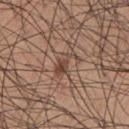Part of a total-body skin-imaging series; this lesion was reviewed on a skin check and was not flagged for biopsy. Imaged with white-light lighting. A region of skin cropped from a whole-body photographic capture, roughly 15 mm wide. The patient is a male aged around 55. Longest diameter approximately 3.5 mm. The lesion is on the left thigh. An algorithmic analysis of the crop reported an area of roughly 5.5 mm², a shape eccentricity near 0.85, and a shape-asymmetry score of about 0.55 (0 = symmetric). And it measured a mean CIELAB color near L≈46 a*≈18 b*≈25, roughly 9 lightness units darker than nearby skin, and a lesion-to-skin contrast of about 7 (normalized; higher = more distinct). And it measured a classifier nevus-likeness of about 85/100.